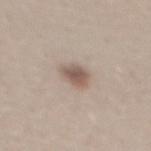This lesion was catalogued during total-body skin photography and was not selected for biopsy. A male patient, aged 28–32. Captured under white-light illumination. The lesion's longest dimension is about 3 mm. The lesion is on the mid back. Cropped from a total-body skin-imaging series; the visible field is about 15 mm.